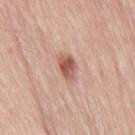Q: Was this lesion biopsied?
A: total-body-photography surveillance lesion; no biopsy
Q: What is the imaging modality?
A: 15 mm crop, total-body photography
Q: Lesion location?
A: the right thigh
Q: What is the lesion's diameter?
A: ≈3 mm
Q: Who is the patient?
A: male, approximately 65 years of age